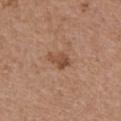Q: Is there a histopathology result?
A: total-body-photography surveillance lesion; no biopsy
Q: How was the tile lit?
A: white-light illumination
Q: How large is the lesion?
A: ≈3 mm
Q: What kind of image is this?
A: total-body-photography crop, ~15 mm field of view
Q: What is the anatomic site?
A: the chest
Q: What are the patient's age and sex?
A: female, approximately 65 years of age